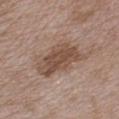<lesion>
<biopsy_status>not biopsied; imaged during a skin examination</biopsy_status>
<lesion_size>
  <long_diameter_mm_approx>6.0</long_diameter_mm_approx>
</lesion_size>
<automated_metrics>
  <area_mm2_approx>14.0</area_mm2_approx>
  <shape_asymmetry>0.2</shape_asymmetry>
  <cielab_L>48</cielab_L>
  <cielab_a>17</cielab_a>
  <cielab_b>26</cielab_b>
  <vs_skin_contrast_norm>8.5</vs_skin_contrast_norm>
  <nevus_likeness_0_100>40</nevus_likeness_0_100>
  <lesion_detection_confidence_0_100>100</lesion_detection_confidence_0_100>
</automated_metrics>
<site>chest</site>
<image>
  <source>total-body photography crop</source>
  <field_of_view_mm>15</field_of_view_mm>
</image>
<lighting>white-light</lighting>
<patient>
  <sex>male</sex>
  <age_approx>50</age_approx>
</patient>
</lesion>